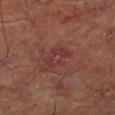{"biopsy_status": "not biopsied; imaged during a skin examination", "patient": {"sex": "male", "age_approx": 65}, "site": "left lower leg", "lesion_size": {"long_diameter_mm_approx": 3.5}, "lighting": "cross-polarized", "image": {"source": "total-body photography crop", "field_of_view_mm": 15}}